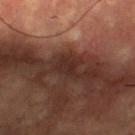Assessment: Part of a total-body skin-imaging series; this lesion was reviewed on a skin check and was not flagged for biopsy. Background: The total-body-photography lesion software estimated an area of roughly 5.5 mm² and two-axis asymmetry of about 0.35. A lesion tile, about 15 mm wide, cut from a 3D total-body photograph. A male subject aged 68–72. The lesion's longest dimension is about 3.5 mm. From the front of the torso. Captured under cross-polarized illumination.